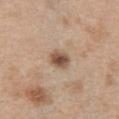biopsy_status: not biopsied; imaged during a skin examination
patient:
  sex: female
  age_approx: 40
image:
  source: total-body photography crop
  field_of_view_mm: 15
site: chest
lesion_size:
  long_diameter_mm_approx: 2.5